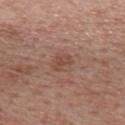This lesion was catalogued during total-body skin photography and was not selected for biopsy. From the back. A lesion tile, about 15 mm wide, cut from a 3D total-body photograph. A female subject aged around 40.